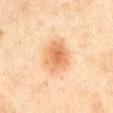biopsy status: no biopsy performed (imaged during a skin exam)
patient: male, in their mid- to late 40s
site: the chest
automated metrics: an area of roughly 11 mm², an outline eccentricity of about 0.8 (0 = round, 1 = elongated), and a shape-asymmetry score of about 0.2 (0 = symmetric); about 10 CIELAB-L* units darker than the surrounding skin and a lesion-to-skin contrast of about 7.5 (normalized; higher = more distinct); a color-variation rating of about 4/10 and radial color variation of about 1
acquisition: 15 mm crop, total-body photography
tile lighting: cross-polarized
diameter: about 5 mm